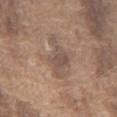{"biopsy_status": "not biopsied; imaged during a skin examination", "patient": {"sex": "male", "age_approx": 75}, "site": "chest", "lighting": "white-light", "automated_metrics": {"area_mm2_approx": 11.0, "shape_asymmetry": 0.35, "color_variation_0_10": 3.0, "peripheral_color_asymmetry": 1.0}, "image": {"source": "total-body photography crop", "field_of_view_mm": 15}}